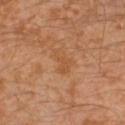Q: Automated lesion metrics?
A: a lesion area of about 4.5 mm², an eccentricity of roughly 0.85, and a shape-asymmetry score of about 0.4 (0 = symmetric); roughly 5 lightness units darker than nearby skin and a normalized lesion–skin contrast near 5; border irregularity of about 4.5 on a 0–10 scale and radial color variation of about 0.5; a nevus-likeness score of about 0/100 and lesion-presence confidence of about 100/100
Q: What are the patient's age and sex?
A: male, aged 28 to 32
Q: How large is the lesion?
A: ~3 mm (longest diameter)
Q: What is the anatomic site?
A: the leg
Q: Illumination type?
A: cross-polarized illumination
Q: What kind of image is this?
A: 15 mm crop, total-body photography